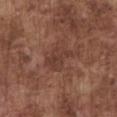<tbp_lesion>
<site>chest</site>
<lesion_size>
  <long_diameter_mm_approx>3.5</long_diameter_mm_approx>
</lesion_size>
<patient>
  <sex>male</sex>
  <age_approx>75</age_approx>
</patient>
<lighting>white-light</lighting>
<image>
  <source>total-body photography crop</source>
  <field_of_view_mm>15</field_of_view_mm>
</image>
</tbp_lesion>